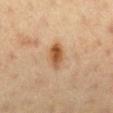This lesion was catalogued during total-body skin photography and was not selected for biopsy.
Cropped from a total-body skin-imaging series; the visible field is about 15 mm.
From the right lower leg.
This is a cross-polarized tile.
A female subject aged around 50.